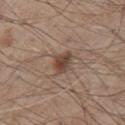{"biopsy_status": "not biopsied; imaged during a skin examination", "lesion_size": {"long_diameter_mm_approx": 3.0}, "lighting": "white-light", "image": {"source": "total-body photography crop", "field_of_view_mm": 15}, "site": "chest", "patient": {"sex": "male", "age_approx": 80}}